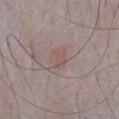Assessment:
Captured during whole-body skin photography for melanoma surveillance; the lesion was not biopsied.
Image and clinical context:
The tile uses white-light illumination. A male subject aged 63 to 67. A 15 mm crop from a total-body photograph taken for skin-cancer surveillance. On the chest. About 2.5 mm across.